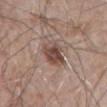Recorded during total-body skin imaging; not selected for excision or biopsy.
The lesion-visualizer software estimated a lesion color around L≈47 a*≈17 b*≈23 in CIELAB. The software also gave border irregularity of about 2.5 on a 0–10 scale and a color-variation rating of about 6/10.
A 15 mm close-up extracted from a 3D total-body photography capture.
Longest diameter approximately 4 mm.
The tile uses white-light illumination.
Located on the abdomen.
The subject is a male aged approximately 80.